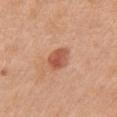Notes:
• biopsy status: total-body-photography surveillance lesion; no biopsy
• imaging modality: ~15 mm tile from a whole-body skin photo
• lesion size: ≈3 mm
• site: the chest
• subject: female, approximately 55 years of age
• illumination: white-light illumination